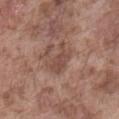Imaged during a routine full-body skin examination; the lesion was not biopsied and no histopathology is available. The patient is a male about 75 years old. The lesion is located on the abdomen. A close-up tile cropped from a whole-body skin photograph, about 15 mm across.